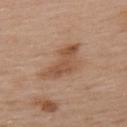This lesion was catalogued during total-body skin photography and was not selected for biopsy. A 15 mm close-up tile from a total-body photography series done for melanoma screening. Measured at roughly 6 mm in maximum diameter. The total-body-photography lesion software estimated a footprint of about 12 mm². The software also gave a border-irregularity rating of about 5/10, a within-lesion color-variation index near 3.5/10, and a peripheral color-asymmetry measure near 1. Captured under white-light illumination. A male patient, aged approximately 55. Located on the upper back.